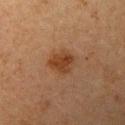  patient:
    sex: female
    age_approx: 60
  lesion_size:
    long_diameter_mm_approx: 3.0
  site: left upper arm
  image:
    source: total-body photography crop
    field_of_view_mm: 15